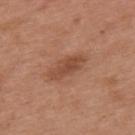| field | value |
|---|---|
| notes | catalogued during a skin exam; not biopsied |
| image-analysis metrics | a lesion area of about 8 mm², a shape eccentricity near 0.9, and a symmetry-axis asymmetry near 0.2; a border-irregularity index near 2.5/10; a classifier nevus-likeness of about 20/100 and lesion-presence confidence of about 100/100 |
| image source | ~15 mm tile from a whole-body skin photo |
| lesion diameter | ~4.5 mm (longest diameter) |
| location | the upper back |
| tile lighting | white-light illumination |
| patient | female, in their 40s |